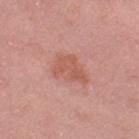The lesion was tiled from a total-body skin photograph and was not biopsied.
Cropped from a whole-body photographic skin survey; the tile spans about 15 mm.
On the right thigh.
Automated image analysis of the tile measured an area of roughly 9.5 mm², a shape eccentricity near 0.75, and two-axis asymmetry of about 0.35.
Imaged with white-light lighting.
The patient is a female aged approximately 40.
Measured at roughly 4 mm in maximum diameter.